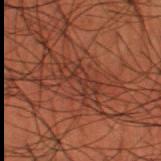Part of a total-body skin-imaging series; this lesion was reviewed on a skin check and was not flagged for biopsy.